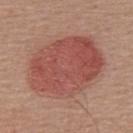This lesion was catalogued during total-body skin photography and was not selected for biopsy. About 9 mm across. A male subject aged 53 to 57. Automated image analysis of the tile measured an average lesion color of about L≈50 a*≈27 b*≈26 (CIELAB) and a normalized lesion–skin contrast near 7. The analysis additionally found a color-variation rating of about 4/10 and peripheral color asymmetry of about 1.5. Imaged with white-light lighting. This image is a 15 mm lesion crop taken from a total-body photograph. From the back.